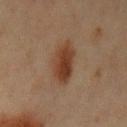Recorded during total-body skin imaging; not selected for excision or biopsy.
The recorded lesion diameter is about 4.5 mm.
Automated image analysis of the tile measured an area of roughly 11 mm², a shape eccentricity near 0.7, and two-axis asymmetry of about 0.2. The analysis additionally found an average lesion color of about L≈34 a*≈18 b*≈27 (CIELAB) and a lesion–skin lightness drop of about 9. And it measured a nevus-likeness score of about 100/100 and a lesion-detection confidence of about 100/100.
A female subject, about 45 years old.
Imaged with cross-polarized lighting.
Located on the left upper arm.
A roughly 15 mm field-of-view crop from a total-body skin photograph.